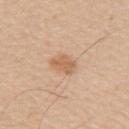{
  "biopsy_status": "not biopsied; imaged during a skin examination",
  "patient": {
    "sex": "male",
    "age_approx": 60
  },
  "lighting": "white-light",
  "site": "arm",
  "image": {
    "source": "total-body photography crop",
    "field_of_view_mm": 15
  }
}